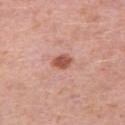Notes:
- notes: catalogued during a skin exam; not biopsied
- diameter: ~2.5 mm (longest diameter)
- tile lighting: white-light
- image source: ~15 mm crop, total-body skin-cancer survey
- patient: female, about 40 years old
- TBP lesion metrics: a lesion area of about 4 mm², an eccentricity of roughly 0.7, and a shape-asymmetry score of about 0.2 (0 = symmetric); an average lesion color of about L≈54 a*≈26 b*≈30 (CIELAB), a lesion–skin lightness drop of about 13, and a lesion-to-skin contrast of about 9 (normalized; higher = more distinct)
- location: the right thigh